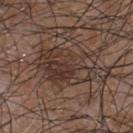Case summary:
– notes: imaged on a skin check; not biopsied
– subject: male, in their mid-50s
– image source: total-body-photography crop, ~15 mm field of view
– image-analysis metrics: two-axis asymmetry of about 0.25; a detector confidence of about 95 out of 100 that the crop contains a lesion
– anatomic site: the chest